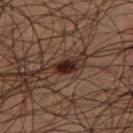<record>
<biopsy_status>not biopsied; imaged during a skin examination</biopsy_status>
<lesion_size>
  <long_diameter_mm_approx>2.5</long_diameter_mm_approx>
</lesion_size>
<site>right thigh</site>
<patient>
  <sex>male</sex>
  <age_approx>50</age_approx>
</patient>
<image>
  <source>total-body photography crop</source>
  <field_of_view_mm>15</field_of_view_mm>
</image>
</record>